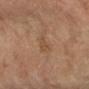The lesion was tiled from a total-body skin photograph and was not biopsied. A roughly 15 mm field-of-view crop from a total-body skin photograph. An algorithmic analysis of the crop reported a footprint of about 3.5 mm², a shape eccentricity near 0.85, and a symmetry-axis asymmetry near 0.5. The analysis additionally found an automated nevus-likeness rating near 0 out of 100. A male subject roughly 60 years of age. Longest diameter approximately 3 mm. The lesion is on the right forearm. This is a cross-polarized tile.